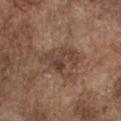Findings:
* notes · total-body-photography surveillance lesion; no biopsy
* image source · total-body-photography crop, ~15 mm field of view
* anatomic site · the chest
* patient · male, aged 73 to 77
* tile lighting · white-light
* image-analysis metrics · a mean CIELAB color near L≈42 a*≈18 b*≈26 and a normalized border contrast of about 7; a border-irregularity index near 6.5/10, a within-lesion color-variation index near 4/10, and a peripheral color-asymmetry measure near 1.5; a nevus-likeness score of about 0/100 and a lesion-detection confidence of about 100/100